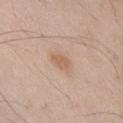Assessment: Captured during whole-body skin photography for melanoma surveillance; the lesion was not biopsied. Background: From the front of the torso. A 15 mm close-up tile from a total-body photography series done for melanoma screening. This is a white-light tile. A male subject, roughly 65 years of age. The lesion's longest dimension is about 3 mm.